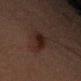No biopsy was performed on this lesion — it was imaged during a full skin examination and was not determined to be concerning.
Cropped from a whole-body photographic skin survey; the tile spans about 15 mm.
On the right upper arm.
The subject is a male in their 50s.
This is a cross-polarized tile.
An algorithmic analysis of the crop reported a footprint of about 6.5 mm² and an eccentricity of roughly 0.75. The analysis additionally found a classifier nevus-likeness of about 90/100 and lesion-presence confidence of about 100/100.
Measured at roughly 3.5 mm in maximum diameter.